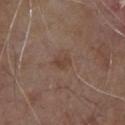Part of a total-body skin-imaging series; this lesion was reviewed on a skin check and was not flagged for biopsy. A 15 mm close-up extracted from a 3D total-body photography capture. The lesion's longest dimension is about 2.5 mm. Located on the chest. The total-body-photography lesion software estimated a lesion area of about 3 mm², an outline eccentricity of about 0.8 (0 = round, 1 = elongated), and a symmetry-axis asymmetry near 0.3. And it measured a lesion color around L≈42 a*≈17 b*≈25 in CIELAB, a lesion–skin lightness drop of about 6, and a normalized lesion–skin contrast near 5.5. The software also gave a border-irregularity index near 3/10 and a within-lesion color-variation index near 1.5/10. Captured under white-light illumination. The subject is a male about 80 years old.